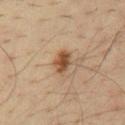No biopsy was performed on this lesion — it was imaged during a full skin examination and was not determined to be concerning.
From the chest.
A region of skin cropped from a whole-body photographic capture, roughly 15 mm wide.
A male patient about 35 years old.